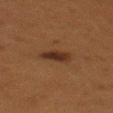Captured during whole-body skin photography for melanoma surveillance; the lesion was not biopsied.